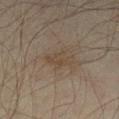<lesion>
<biopsy_status>not biopsied; imaged during a skin examination</biopsy_status>
<automated_metrics>
  <vs_skin_darker_L>5.0</vs_skin_darker_L>
  <vs_skin_contrast_norm>5.0</vs_skin_contrast_norm>
  <nevus_likeness_0_100>0</nevus_likeness_0_100>
</automated_metrics>
<lighting>cross-polarized</lighting>
<site>left lower leg</site>
<patient>
  <sex>male</sex>
  <age_approx>45</age_approx>
</patient>
<image>
  <source>total-body photography crop</source>
  <field_of_view_mm>15</field_of_view_mm>
</image>
</lesion>